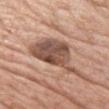This lesion was catalogued during total-body skin photography and was not selected for biopsy.